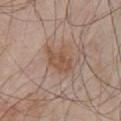Assessment:
The lesion was photographed on a routine skin check and not biopsied; there is no pathology result.
Acquisition and patient details:
Cropped from a whole-body photographic skin survey; the tile spans about 15 mm. A male patient, about 55 years old. The lesion is on the chest. This is a white-light tile. An algorithmic analysis of the crop reported a border-irregularity rating of about 3.5/10 and internal color variation of about 2.5 on a 0–10 scale. The analysis additionally found a lesion-detection confidence of about 100/100.